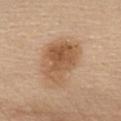Q: Was this lesion biopsied?
A: total-body-photography surveillance lesion; no biopsy
Q: What lighting was used for the tile?
A: white-light illumination
Q: What is the anatomic site?
A: the chest
Q: What is the imaging modality?
A: ~15 mm tile from a whole-body skin photo
Q: Patient demographics?
A: female, aged 63 to 67
Q: How large is the lesion?
A: ≈5.5 mm